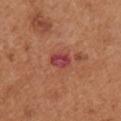A female subject in their mid- to late 60s. A region of skin cropped from a whole-body photographic capture, roughly 15 mm wide. The lesion-visualizer software estimated a lesion color around L≈43 a*≈35 b*≈25 in CIELAB, about 10 CIELAB-L* units darker than the surrounding skin, and a normalized lesion–skin contrast near 10. The analysis additionally found a border-irregularity index near 2/10. The lesion is on the right upper arm.